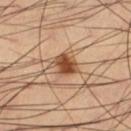notes: no biopsy performed (imaged during a skin exam)
size: ≈3 mm
automated lesion analysis: a classifier nevus-likeness of about 100/100 and a lesion-detection confidence of about 100/100
site: the left thigh
acquisition: total-body-photography crop, ~15 mm field of view
patient: male, approximately 65 years of age
tile lighting: cross-polarized